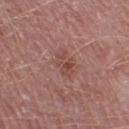Assessment:
This lesion was catalogued during total-body skin photography and was not selected for biopsy.
Clinical summary:
Imaged with white-light lighting. On the left thigh. A male subject, aged around 55. This image is a 15 mm lesion crop taken from a total-body photograph. Automated image analysis of the tile measured about 7 CIELAB-L* units darker than the surrounding skin and a normalized lesion–skin contrast near 6. The analysis additionally found a border-irregularity index near 4.5/10, a color-variation rating of about 2.5/10, and radial color variation of about 1.